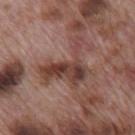biopsy_status: not biopsied; imaged during a skin examination
patient:
  sex: male
  age_approx: 70
lesion_size:
  long_diameter_mm_approx: 6.5
site: mid back
image:
  source: total-body photography crop
  field_of_view_mm: 15